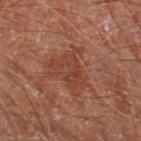The lesion was photographed on a routine skin check and not biopsied; there is no pathology result. The subject is a male aged 68 to 72. The lesion is on the left thigh. Imaged with cross-polarized lighting. The lesion-visualizer software estimated a peripheral color-asymmetry measure near 1. The lesion's longest dimension is about 5 mm. A lesion tile, about 15 mm wide, cut from a 3D total-body photograph.